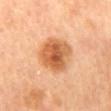Clinical impression:
The lesion was tiled from a total-body skin photograph and was not biopsied.
Image and clinical context:
The tile uses cross-polarized illumination. The lesion is located on the mid back. A region of skin cropped from a whole-body photographic capture, roughly 15 mm wide. A female patient, in their mid- to late 60s.